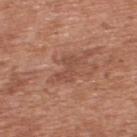biopsy status: imaged on a skin check; not biopsied | TBP lesion metrics: a footprint of about 4.5 mm², a shape eccentricity near 0.85, and two-axis asymmetry of about 0.4; a normalized border contrast of about 5; border irregularity of about 5.5 on a 0–10 scale, internal color variation of about 0 on a 0–10 scale, and peripheral color asymmetry of about 0; a detector confidence of about 100 out of 100 that the crop contains a lesion | image source: ~15 mm tile from a whole-body skin photo | lighting: white-light | body site: the upper back | lesion diameter: ~3 mm (longest diameter) | subject: male, approximately 70 years of age.